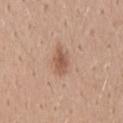Clinical impression: The lesion was photographed on a routine skin check and not biopsied; there is no pathology result. Clinical summary: A close-up tile cropped from a whole-body skin photograph, about 15 mm across. Automated tile analysis of the lesion measured an area of roughly 5 mm² and a symmetry-axis asymmetry near 0.25. The analysis additionally found border irregularity of about 3 on a 0–10 scale, a within-lesion color-variation index near 2/10, and peripheral color asymmetry of about 0.5. The lesion is located on the mid back. A male subject, about 40 years old.